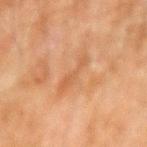Recorded during total-body skin imaging; not selected for excision or biopsy. Cropped from a whole-body photographic skin survey; the tile spans about 15 mm. An algorithmic analysis of the crop reported an outline eccentricity of about 0.95 (0 = round, 1 = elongated) and a shape-asymmetry score of about 0.35 (0 = symmetric). The recorded lesion diameter is about 5 mm. A male subject roughly 45 years of age. The tile uses cross-polarized illumination. The lesion is located on the right forearm.